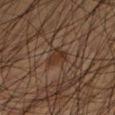Recorded during total-body skin imaging; not selected for excision or biopsy. The lesion's longest dimension is about 2.5 mm. A male subject, aged approximately 45. A roughly 15 mm field-of-view crop from a total-body skin photograph. The lesion is on the left lower leg. Imaged with cross-polarized lighting.